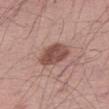follow-up: imaged on a skin check; not biopsied
illumination: white-light illumination
automated lesion analysis: an average lesion color of about L≈49 a*≈21 b*≈24 (CIELAB), a lesion–skin lightness drop of about 13, and a lesion-to-skin contrast of about 9 (normalized; higher = more distinct)
lesion size: about 4 mm
subject: male, roughly 65 years of age
image: ~15 mm crop, total-body skin-cancer survey
body site: the right thigh